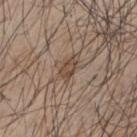Impression: Recorded during total-body skin imaging; not selected for excision or biopsy. Context: The tile uses white-light illumination. A male patient about 45 years old. A 15 mm crop from a total-body photograph taken for skin-cancer surveillance. The lesion is located on the back. Measured at roughly 2.5 mm in maximum diameter.